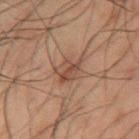The lesion was tiled from a total-body skin photograph and was not biopsied. Automated tile analysis of the lesion measured a footprint of about 7.5 mm², a shape eccentricity near 0.55, and a symmetry-axis asymmetry near 0.3. It also reported a lesion color around L≈36 a*≈15 b*≈22 in CIELAB, a lesion–skin lightness drop of about 7, and a normalized border contrast of about 6.5. The analysis additionally found a border-irregularity index near 3.5/10 and a within-lesion color-variation index near 4/10. A male subject, aged 48–52. A roughly 15 mm field-of-view crop from a total-body skin photograph. The lesion is located on the mid back. Imaged with cross-polarized lighting.